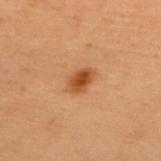* follow-up: catalogued during a skin exam; not biopsied
* patient: female, aged around 40
* tile lighting: cross-polarized illumination
* automated metrics: a lesion–skin lightness drop of about 11 and a lesion-to-skin contrast of about 9 (normalized; higher = more distinct); a lesion-detection confidence of about 100/100
* acquisition: ~15 mm tile from a whole-body skin photo
* anatomic site: the upper back